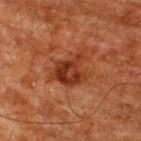notes: no biopsy performed (imaged during a skin exam) | image source: ~15 mm tile from a whole-body skin photo | location: the upper back | image-analysis metrics: an eccentricity of roughly 0.65 and a symmetry-axis asymmetry near 0.45; a border-irregularity index near 5.5/10 and a color-variation rating of about 5/10 | diameter: ≈5 mm | lighting: cross-polarized | patient: male, roughly 60 years of age.